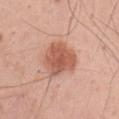biopsy status: catalogued during a skin exam; not biopsied | image source: total-body-photography crop, ~15 mm field of view | lesion size: ≈5.5 mm | site: the left upper arm | tile lighting: white-light | image-analysis metrics: an area of roughly 14 mm², an outline eccentricity of about 0.7 (0 = round, 1 = elongated), and a symmetry-axis asymmetry near 0.25; a lesion color around L≈58 a*≈25 b*≈31 in CIELAB, about 13 CIELAB-L* units darker than the surrounding skin, and a normalized lesion–skin contrast near 8.5; a border-irregularity index near 2.5/10, a color-variation rating of about 3/10, and peripheral color asymmetry of about 1; a nevus-likeness score of about 100/100 and a detector confidence of about 100 out of 100 that the crop contains a lesion | patient: male, roughly 55 years of age.